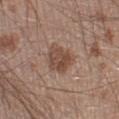Recorded during total-body skin imaging; not selected for excision or biopsy. Imaged with white-light lighting. On the left lower leg. This image is a 15 mm lesion crop taken from a total-body photograph. The total-body-photography lesion software estimated an average lesion color of about L≈47 a*≈19 b*≈26 (CIELAB). The analysis additionally found border irregularity of about 3 on a 0–10 scale, a within-lesion color-variation index near 4/10, and peripheral color asymmetry of about 1.5. And it measured a lesion-detection confidence of about 100/100. Measured at roughly 3.5 mm in maximum diameter. A male subject aged 18 to 22.